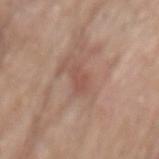Clinical summary: About 3 mm across. A male subject in their 80s. Imaged with white-light lighting. Automated image analysis of the tile measured a lesion area of about 3.5 mm² and an outline eccentricity of about 0.9 (0 = round, 1 = elongated). And it measured a border-irregularity index near 3.5/10 and internal color variation of about 0.5 on a 0–10 scale. And it measured a classifier nevus-likeness of about 0/100. The lesion is on the mid back. A 15 mm close-up tile from a total-body photography series done for melanoma screening.